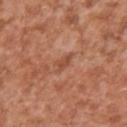Findings:
– workup: total-body-photography surveillance lesion; no biopsy
– acquisition: ~15 mm tile from a whole-body skin photo
– illumination: white-light illumination
– body site: the right upper arm
– subject: male, aged approximately 45
– lesion diameter: about 2.5 mm